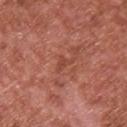| key | value |
|---|---|
| workup | catalogued during a skin exam; not biopsied |
| diameter | ≈3 mm |
| subject | male, approximately 65 years of age |
| location | the back |
| imaging modality | 15 mm crop, total-body photography |
| lighting | white-light |